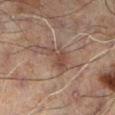Notes:
- workup — total-body-photography surveillance lesion; no biopsy
- location — the left lower leg
- illumination — cross-polarized
- patient — male, aged 58 to 62
- lesion size — about 4 mm
- acquisition — ~15 mm tile from a whole-body skin photo
- TBP lesion metrics — a border-irregularity rating of about 5.5/10, a color-variation rating of about 3.5/10, and radial color variation of about 1.5; a nevus-likeness score of about 15/100 and a lesion-detection confidence of about 100/100